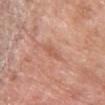Clinical impression: No biopsy was performed on this lesion — it was imaged during a full skin examination and was not determined to be concerning.